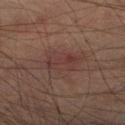Clinical impression:
No biopsy was performed on this lesion — it was imaged during a full skin examination and was not determined to be concerning.